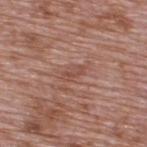Imaged during a routine full-body skin examination; the lesion was not biopsied and no histopathology is available. A 15 mm crop from a total-body photograph taken for skin-cancer surveillance. The patient is a male aged around 70. From the upper back. Measured at roughly 2.5 mm in maximum diameter.